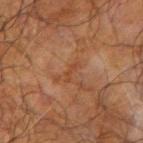notes: catalogued during a skin exam; not biopsied | patient: male, approximately 70 years of age | tile lighting: cross-polarized illumination | anatomic site: the left forearm | image source: total-body-photography crop, ~15 mm field of view | diameter: about 2.5 mm.